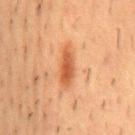Impression: This lesion was catalogued during total-body skin photography and was not selected for biopsy. Background: A male subject, aged approximately 55. Automated image analysis of the tile measured an area of roughly 7 mm² and an eccentricity of roughly 0.95. A region of skin cropped from a whole-body photographic capture, roughly 15 mm wide. Captured under cross-polarized illumination. Measured at roughly 5 mm in maximum diameter. The lesion is located on the mid back.